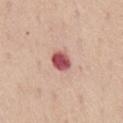workup: total-body-photography surveillance lesion; no biopsy